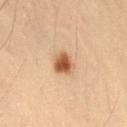Notes:
- workup — imaged on a skin check; not biopsied
- image source — ~15 mm crop, total-body skin-cancer survey
- tile lighting — cross-polarized
- subject — male, in their mid- to late 50s
- lesion diameter — ≈2.5 mm
- location — the front of the torso